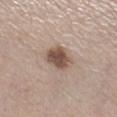<record>
  <biopsy_status>not biopsied; imaged during a skin examination</biopsy_status>
  <patient>
    <sex>female</sex>
    <age_approx>60</age_approx>
  </patient>
  <lighting>white-light</lighting>
  <lesion_size>
    <long_diameter_mm_approx>4.0</long_diameter_mm_approx>
  </lesion_size>
  <image>
    <source>total-body photography crop</source>
    <field_of_view_mm>15</field_of_view_mm>
  </image>
  <automated_metrics>
    <vs_skin_darker_L>14.0</vs_skin_darker_L>
    <border_irregularity_0_10>2.0</border_irregularity_0_10>
    <color_variation_0_10>3.5</color_variation_0_10>
    <peripheral_color_asymmetry>1.0</peripheral_color_asymmetry>
    <nevus_likeness_0_100>90</nevus_likeness_0_100>
    <lesion_detection_confidence_0_100>100</lesion_detection_confidence_0_100>
  </automated_metrics>
  <site>right lower leg</site>
</record>